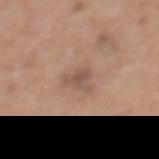subject = female, roughly 60 years of age
automated metrics = an eccentricity of roughly 0.75 and a symmetry-axis asymmetry near 0.5; a within-lesion color-variation index near 2/10 and radial color variation of about 0.5; an automated nevus-likeness rating near 0 out of 100 and a detector confidence of about 100 out of 100 that the crop contains a lesion
diameter = about 3.5 mm
site = the upper back
acquisition = ~15 mm crop, total-body skin-cancer survey